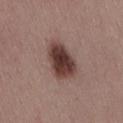No biopsy was performed on this lesion — it was imaged during a full skin examination and was not determined to be concerning. The lesion-visualizer software estimated a footprint of about 12 mm², an outline eccentricity of about 0.75 (0 = round, 1 = elongated), and a shape-asymmetry score of about 0.2 (0 = symmetric). The software also gave an average lesion color of about L≈39 a*≈19 b*≈21 (CIELAB), about 16 CIELAB-L* units darker than the surrounding skin, and a lesion-to-skin contrast of about 12.5 (normalized; higher = more distinct). The software also gave a nevus-likeness score of about 100/100 and a detector confidence of about 100 out of 100 that the crop contains a lesion. About 4.5 mm across. A female patient, in their mid- to late 50s. The lesion is on the back. A close-up tile cropped from a whole-body skin photograph, about 15 mm across. Imaged with white-light lighting.